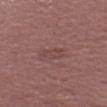Impression:
Recorded during total-body skin imaging; not selected for excision or biopsy.
Image and clinical context:
The patient is a female roughly 45 years of age. Approximately 3 mm at its widest. A roughly 15 mm field-of-view crop from a total-body skin photograph. On the head or neck.